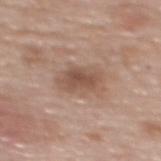notes: no biopsy performed (imaged during a skin exam) | subject: female, aged around 60 | automated lesion analysis: a footprint of about 10 mm², an eccentricity of roughly 0.85, and two-axis asymmetry of about 0.2; roughly 10 lightness units darker than nearby skin; a border-irregularity index near 3/10, internal color variation of about 3 on a 0–10 scale, and radial color variation of about 1; a classifier nevus-likeness of about 35/100 and lesion-presence confidence of about 100/100 | imaging modality: ~15 mm tile from a whole-body skin photo | size: ~5 mm (longest diameter) | anatomic site: the upper back.